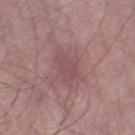This lesion was catalogued during total-body skin photography and was not selected for biopsy. Cropped from a whole-body photographic skin survey; the tile spans about 15 mm. The lesion's longest dimension is about 4 mm. The lesion is located on the leg. The total-body-photography lesion software estimated a lesion color around L≈49 a*≈21 b*≈17 in CIELAB, roughly 6 lightness units darker than nearby skin, and a normalized border contrast of about 5. And it measured an automated nevus-likeness rating near 0 out of 100 and a detector confidence of about 75 out of 100 that the crop contains a lesion. A male subject in their mid- to late 60s.